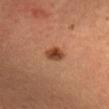<record>
<biopsy_status>not biopsied; imaged during a skin examination</biopsy_status>
<patient>
  <sex>male</sex>
  <age_approx>20</age_approx>
</patient>
<site>head or neck</site>
<lighting>cross-polarized</lighting>
<automated_metrics>
  <color_variation_0_10>4.0</color_variation_0_10>
  <peripheral_color_asymmetry>1.0</peripheral_color_asymmetry>
</automated_metrics>
<image>
  <source>total-body photography crop</source>
  <field_of_view_mm>15</field_of_view_mm>
</image>
</record>